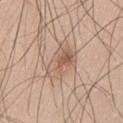Q: Was this lesion biopsied?
A: no biopsy performed (imaged during a skin exam)
Q: Lesion location?
A: the leg
Q: What is the imaging modality?
A: ~15 mm crop, total-body skin-cancer survey
Q: How large is the lesion?
A: ≈5 mm
Q: Patient demographics?
A: male, aged approximately 60
Q: Automated lesion metrics?
A: an average lesion color of about L≈59 a*≈17 b*≈28 (CIELAB), a lesion–skin lightness drop of about 9, and a lesion-to-skin contrast of about 6 (normalized; higher = more distinct); a nevus-likeness score of about 5/100 and a lesion-detection confidence of about 100/100
Q: Illumination type?
A: white-light illumination